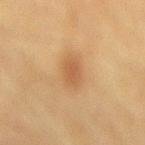Findings:
– workup · catalogued during a skin exam; not biopsied
– lesion size · ≈4 mm
– location · the mid back
– image · ~15 mm tile from a whole-body skin photo
– automated metrics · a footprint of about 7.5 mm² and an eccentricity of roughly 0.85; a lesion-detection confidence of about 100/100
– subject · female, in their mid- to late 50s
– tile lighting · cross-polarized illumination